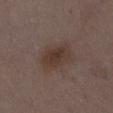Q: Who is the patient?
A: female, aged 33–37
Q: What did automated image analysis measure?
A: an area of roughly 10 mm² and two-axis asymmetry of about 0.15; an automated nevus-likeness rating near 60 out of 100 and a detector confidence of about 100 out of 100 that the crop contains a lesion
Q: How was this image acquired?
A: ~15 mm crop, total-body skin-cancer survey
Q: Where on the body is the lesion?
A: the right thigh